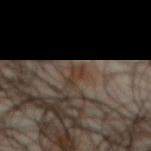Imaged during a routine full-body skin examination; the lesion was not biopsied and no histopathology is available.
Longest diameter approximately 2.5 mm.
A male subject, aged 63–67.
A close-up tile cropped from a whole-body skin photograph, about 15 mm across.
The lesion is on the chest.